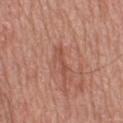Assessment: No biopsy was performed on this lesion — it was imaged during a full skin examination and was not determined to be concerning. Background: Cropped from a total-body skin-imaging series; the visible field is about 15 mm. The lesion is located on the upper back. A male subject, aged 68 to 72. The total-body-photography lesion software estimated a lesion area of about 3.5 mm², an eccentricity of roughly 0.95, and two-axis asymmetry of about 0.35. The software also gave a mean CIELAB color near L≈51 a*≈26 b*≈29 and a normalized lesion–skin contrast near 5.5.